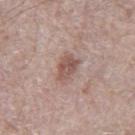<lesion>
  <image>
    <source>total-body photography crop</source>
    <field_of_view_mm>15</field_of_view_mm>
  </image>
  <automated_metrics>
    <border_irregularity_0_10>3.0</border_irregularity_0_10>
    <color_variation_0_10>3.0</color_variation_0_10>
    <peripheral_color_asymmetry>1.0</peripheral_color_asymmetry>
    <lesion_detection_confidence_0_100>100</lesion_detection_confidence_0_100>
  </automated_metrics>
  <patient>
    <sex>male</sex>
    <age_approx>70</age_approx>
  </patient>
  <site>right thigh</site>
  <lighting>white-light</lighting>
</lesion>